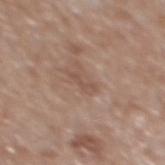The lesion was tiled from a total-body skin photograph and was not biopsied. Automated image analysis of the tile measured an average lesion color of about L≈51 a*≈18 b*≈26 (CIELAB) and a normalized border contrast of about 5. The software also gave border irregularity of about 7 on a 0–10 scale and radial color variation of about 0. And it measured a detector confidence of about 100 out of 100 that the crop contains a lesion. A close-up tile cropped from a whole-body skin photograph, about 15 mm across. On the mid back. A male patient, in their mid-60s. The tile uses white-light illumination.